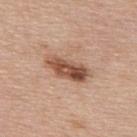Q: Was this lesion biopsied?
A: catalogued during a skin exam; not biopsied
Q: How was this image acquired?
A: ~15 mm tile from a whole-body skin photo
Q: What are the patient's age and sex?
A: male, aged 73–77
Q: Lesion location?
A: the upper back
Q: What is the lesion's diameter?
A: about 5.5 mm
Q: Illumination type?
A: white-light illumination